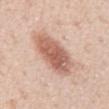Imaged during a routine full-body skin examination; the lesion was not biopsied and no histopathology is available.
Cropped from a whole-body photographic skin survey; the tile spans about 15 mm.
A male patient in their mid-50s.
From the abdomen.